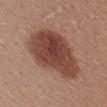workup = catalogued during a skin exam; not biopsied
diameter = about 9 mm
image-analysis metrics = a lesion color around L≈44 a*≈23 b*≈26 in CIELAB and a normalized border contrast of about 10.5; border irregularity of about 2.5 on a 0–10 scale and peripheral color asymmetry of about 1.5; an automated nevus-likeness rating near 95 out of 100 and a detector confidence of about 100 out of 100 that the crop contains a lesion
subject = female, about 25 years old
anatomic site = the mid back
lighting = white-light
acquisition = ~15 mm tile from a whole-body skin photo